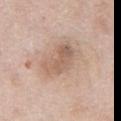This lesion was catalogued during total-body skin photography and was not selected for biopsy.
A 15 mm close-up extracted from a 3D total-body photography capture.
The subject is a male roughly 75 years of age.
Located on the abdomen.